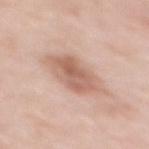Clinical impression: The lesion was tiled from a total-body skin photograph and was not biopsied. Acquisition and patient details: A 15 mm crop from a total-body photograph taken for skin-cancer surveillance. A female patient roughly 40 years of age. The lesion-visualizer software estimated a lesion area of about 16 mm² and two-axis asymmetry of about 0.25. The software also gave a mean CIELAB color near L≈62 a*≈20 b*≈28, about 11 CIELAB-L* units darker than the surrounding skin, and a normalized lesion–skin contrast near 7. It also reported a nevus-likeness score of about 55/100. The tile uses white-light illumination. On the mid back. The lesion's longest dimension is about 6 mm.